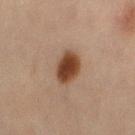  biopsy_status: not biopsied; imaged during a skin examination
  site: right thigh
  lesion_size:
    long_diameter_mm_approx: 4.0
  patient:
    sex: female
    age_approx: 45
  image:
    source: total-body photography crop
    field_of_view_mm: 15